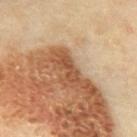  biopsy_status: not biopsied; imaged during a skin examination
  patient:
    sex: male
    age_approx: 70
  image:
    source: total-body photography crop
    field_of_view_mm: 15
  site: abdomen